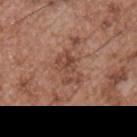Assessment: Imaged during a routine full-body skin examination; the lesion was not biopsied and no histopathology is available. Image and clinical context: The lesion's longest dimension is about 4 mm. A region of skin cropped from a whole-body photographic capture, roughly 15 mm wide. The subject is a male aged 53 to 57. The tile uses white-light illumination. From the chest.